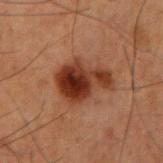Background: A male patient, aged around 60. A region of skin cropped from a whole-body photographic capture, roughly 15 mm wide. The recorded lesion diameter is about 5.5 mm. Imaged with cross-polarized lighting. The total-body-photography lesion software estimated a mean CIELAB color near L≈27 a*≈21 b*≈25, a lesion–skin lightness drop of about 12, and a lesion-to-skin contrast of about 12 (normalized; higher = more distinct). It also reported a border-irregularity rating of about 4/10, a within-lesion color-variation index near 7.5/10, and a peripheral color-asymmetry measure near 2.5. The analysis additionally found a nevus-likeness score of about 100/100 and a detector confidence of about 100 out of 100 that the crop contains a lesion. Located on the right upper arm.